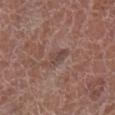Imaged during a routine full-body skin examination; the lesion was not biopsied and no histopathology is available.
The lesion is located on the right lower leg.
A 15 mm close-up tile from a total-body photography series done for melanoma screening.
Captured under white-light illumination.
A male patient aged around 75.